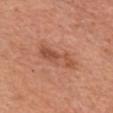workup: total-body-photography surveillance lesion; no biopsy | automated lesion analysis: an eccentricity of roughly 0.95 and a shape-asymmetry score of about 0.45 (0 = symmetric); an average lesion color of about L≈52 a*≈26 b*≈33 (CIELAB), about 10 CIELAB-L* units darker than the surrounding skin, and a normalized border contrast of about 6.5; border irregularity of about 6.5 on a 0–10 scale, internal color variation of about 2.5 on a 0–10 scale, and radial color variation of about 1 | location: the chest | subject: female, aged 58 to 62 | acquisition: total-body-photography crop, ~15 mm field of view | lighting: white-light.